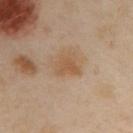Located on the left arm.
This image is a 15 mm lesion crop taken from a total-body photograph.
A female subject, about 40 years old.